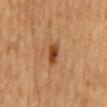<case>
<biopsy_status>not biopsied; imaged during a skin examination</biopsy_status>
<site>mid back</site>
<image>
  <source>total-body photography crop</source>
  <field_of_view_mm>15</field_of_view_mm>
</image>
<patient>
  <sex>male</sex>
  <age_approx>65</age_approx>
</patient>
<lighting>cross-polarized</lighting>
<lesion_size>
  <long_diameter_mm_approx>3.0</long_diameter_mm_approx>
</lesion_size>
</case>